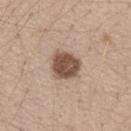<record>
<biopsy_status>not biopsied; imaged during a skin examination</biopsy_status>
<lesion_size>
  <long_diameter_mm_approx>3.5</long_diameter_mm_approx>
</lesion_size>
<patient>
  <sex>female</sex>
  <age_approx>40</age_approx>
</patient>
<image>
  <source>total-body photography crop</source>
  <field_of_view_mm>15</field_of_view_mm>
</image>
<site>arm</site>
<automated_metrics>
  <border_irregularity_0_10>2.5</border_irregularity_0_10>
  <color_variation_0_10>3.5</color_variation_0_10>
  <peripheral_color_asymmetry>1.5</peripheral_color_asymmetry>
  <nevus_likeness_0_100>90</nevus_likeness_0_100>
</automated_metrics>
<lighting>white-light</lighting>
</record>